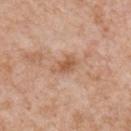Assessment: Part of a total-body skin-imaging series; this lesion was reviewed on a skin check and was not flagged for biopsy. Acquisition and patient details: The subject is a male about 65 years old. The lesion-visualizer software estimated a mean CIELAB color near L≈58 a*≈21 b*≈33, roughly 9 lightness units darker than nearby skin, and a normalized lesion–skin contrast near 6.5. The lesion is located on the chest. A lesion tile, about 15 mm wide, cut from a 3D total-body photograph.